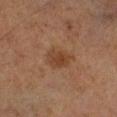No biopsy was performed on this lesion — it was imaged during a full skin examination and was not determined to be concerning. The patient is a male in their mid-60s. This is a cross-polarized tile. A 15 mm close-up tile from a total-body photography series done for melanoma screening. Located on the right lower leg. Approximately 3.5 mm at its widest.